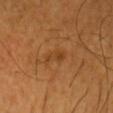The lesion was tiled from a total-body skin photograph and was not biopsied. An algorithmic analysis of the crop reported a footprint of about 3.5 mm² and two-axis asymmetry of about 0.3. It also reported a mean CIELAB color near L≈41 a*≈23 b*≈39, a lesion–skin lightness drop of about 6, and a lesion-to-skin contrast of about 5.5 (normalized; higher = more distinct). The software also gave border irregularity of about 3.5 on a 0–10 scale, internal color variation of about 1.5 on a 0–10 scale, and peripheral color asymmetry of about 0.5. And it measured an automated nevus-likeness rating near 5 out of 100 and a detector confidence of about 100 out of 100 that the crop contains a lesion. The tile uses cross-polarized illumination. Located on the head or neck. Cropped from a total-body skin-imaging series; the visible field is about 15 mm. The subject is a male aged 58 to 62.